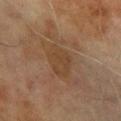subject = male, in their 70s; automated metrics = a lesion–skin lightness drop of about 5 and a normalized border contrast of about 5; lighting = cross-polarized; image = ~15 mm tile from a whole-body skin photo; body site = the left forearm; size = ≈4 mm.